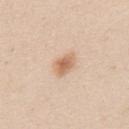  biopsy_status: not biopsied; imaged during a skin examination
  site: upper back
  lighting: white-light
  image:
    source: total-body photography crop
    field_of_view_mm: 15
  lesion_size:
    long_diameter_mm_approx: 3.0
  patient:
    sex: male
    age_approx: 25